Located on the left lower leg. Longest diameter approximately 3 mm. This image is a 15 mm lesion crop taken from a total-body photograph. Imaged with white-light lighting. A female patient aged around 25.
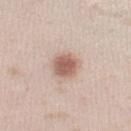Diagnosis:
The lesion was biopsied, and histopathology showed a dysplastic (Clark) nevus.